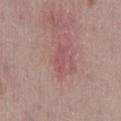Clinical summary:
Longest diameter approximately 1.5 mm. The patient is a female aged 48–52. The total-body-photography lesion software estimated a border-irregularity rating of about 2.5/10 and a within-lesion color-variation index near 0/10. This is a white-light tile. Located on the mid back. A 15 mm close-up extracted from a 3D total-body photography capture.
Conclusion:
Biopsy histopathology demonstrated a superficial basal cell carcinoma, classified as a malignancy.